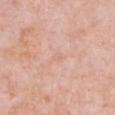biopsy status: imaged on a skin check; not biopsied | site: the chest | acquisition: ~15 mm tile from a whole-body skin photo | TBP lesion metrics: an area of roughly 1 mm² and a shape-asymmetry score of about 0.3 (0 = symmetric); a classifier nevus-likeness of about 0/100 | subject: female, roughly 50 years of age.